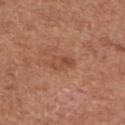No biopsy was performed on this lesion — it was imaged during a full skin examination and was not determined to be concerning. A 15 mm close-up extracted from a 3D total-body photography capture. The lesion is on the left upper arm. The lesion's longest dimension is about 3 mm. The patient is a female aged approximately 80. Captured under white-light illumination. Automated image analysis of the tile measured a mean CIELAB color near L≈48 a*≈24 b*≈31 and about 8 CIELAB-L* units darker than the surrounding skin.